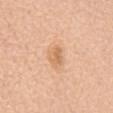<case>
<biopsy_status>not biopsied; imaged during a skin examination</biopsy_status>
<lesion_size>
  <long_diameter_mm_approx>3.0</long_diameter_mm_approx>
</lesion_size>
<patient>
  <sex>female</sex>
  <age_approx>70</age_approx>
</patient>
<lighting>white-light</lighting>
<image>
  <source>total-body photography crop</source>
  <field_of_view_mm>15</field_of_view_mm>
</image>
<site>mid back</site>
</case>